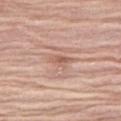Acquisition and patient details:
Located on the right thigh. A close-up tile cropped from a whole-body skin photograph, about 15 mm across. The lesion-visualizer software estimated an area of roughly 3 mm² and two-axis asymmetry of about 0.35. It also reported a nevus-likeness score of about 0/100 and a lesion-detection confidence of about 70/100. The lesion's longest dimension is about 2.5 mm. A female subject, aged around 75.